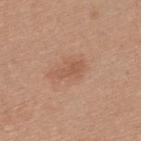Assessment: The lesion was tiled from a total-body skin photograph and was not biopsied. Context: A lesion tile, about 15 mm wide, cut from a 3D total-body photograph. The total-body-photography lesion software estimated an average lesion color of about L≈55 a*≈22 b*≈31 (CIELAB), about 7 CIELAB-L* units darker than the surrounding skin, and a lesion-to-skin contrast of about 5 (normalized; higher = more distinct). It also reported a border-irregularity rating of about 3/10, internal color variation of about 1.5 on a 0–10 scale, and radial color variation of about 0.5. The analysis additionally found an automated nevus-likeness rating near 55 out of 100. Captured under white-light illumination. Measured at roughly 3.5 mm in maximum diameter. A male subject aged 28–32. Located on the upper back.